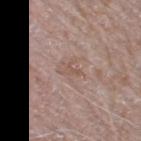{
  "biopsy_status": "not biopsied; imaged during a skin examination",
  "patient": {
    "sex": "male",
    "age_approx": 75
  },
  "site": "right thigh",
  "lighting": "white-light",
  "image": {
    "source": "total-body photography crop",
    "field_of_view_mm": 15
  },
  "lesion_size": {
    "long_diameter_mm_approx": 2.5
  },
  "automated_metrics": {
    "cielab_L": 54,
    "cielab_a": 17,
    "cielab_b": 24,
    "vs_skin_darker_L": 6.0,
    "vs_skin_contrast_norm": 5.0
  }
}